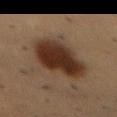Notes:
* workup: total-body-photography surveillance lesion; no biopsy
* location: the mid back
* illumination: cross-polarized
* automated lesion analysis: a mean CIELAB color near L≈27 a*≈15 b*≈23, about 13 CIELAB-L* units darker than the surrounding skin, and a normalized lesion–skin contrast near 12.5; internal color variation of about 4.5 on a 0–10 scale and peripheral color asymmetry of about 1.5; an automated nevus-likeness rating near 100 out of 100 and a lesion-detection confidence of about 100/100
* patient: male, approximately 55 years of age
* image source: ~15 mm tile from a whole-body skin photo